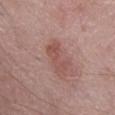Recorded during total-body skin imaging; not selected for excision or biopsy. A roughly 15 mm field-of-view crop from a total-body skin photograph. A male subject, aged around 70. From the abdomen. This is a white-light tile.